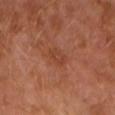Part of a total-body skin-imaging series; this lesion was reviewed on a skin check and was not flagged for biopsy. The tile uses cross-polarized illumination. The subject is a male in their 30s. The lesion is on the left upper arm. Automated tile analysis of the lesion measured a nevus-likeness score of about 0/100. Measured at roughly 2.5 mm in maximum diameter. A 15 mm close-up tile from a total-body photography series done for melanoma screening.